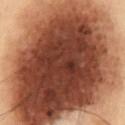Captured during whole-body skin photography for melanoma surveillance; the lesion was not biopsied. Longest diameter approximately 15.5 mm. A male patient aged around 55. From the abdomen. A 15 mm close-up tile from a total-body photography series done for melanoma screening. The total-body-photography lesion software estimated an outline eccentricity of about 0.55 (0 = round, 1 = elongated) and two-axis asymmetry of about 0.15. The software also gave an average lesion color of about L≈37 a*≈20 b*≈26 (CIELAB) and a lesion-to-skin contrast of about 16 (normalized; higher = more distinct). And it measured a border-irregularity index near 2/10, a within-lesion color-variation index near 9.5/10, and radial color variation of about 3. It also reported an automated nevus-likeness rating near 90 out of 100 and lesion-presence confidence of about 100/100. This is a cross-polarized tile.